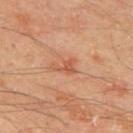Q: Is there a histopathology result?
A: total-body-photography surveillance lesion; no biopsy
Q: What is the imaging modality?
A: ~15 mm tile from a whole-body skin photo
Q: Lesion size?
A: ≈3 mm
Q: Who is the patient?
A: male, aged around 65
Q: What did automated image analysis measure?
A: an eccentricity of roughly 0.75 and a symmetry-axis asymmetry near 0.6; border irregularity of about 6.5 on a 0–10 scale, internal color variation of about 1 on a 0–10 scale, and a peripheral color-asymmetry measure near 0
Q: Where on the body is the lesion?
A: the upper back
Q: Illumination type?
A: cross-polarized